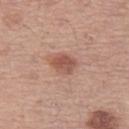  biopsy_status: not biopsied; imaged during a skin examination
  patient:
    sex: female
    age_approx: 50
  image:
    source: total-body photography crop
    field_of_view_mm: 15
  lesion_size:
    long_diameter_mm_approx: 3.0
  lighting: white-light
  automated_metrics:
    shape_asymmetry: 0.25
    cielab_L: 53
    cielab_a: 23
    cielab_b: 28
    vs_skin_darker_L: 11.0
    color_variation_0_10: 3.5
    peripheral_color_asymmetry: 1.5
    nevus_likeness_0_100: 95
    lesion_detection_confidence_0_100: 100
  site: left thigh